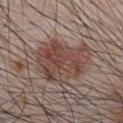No biopsy was performed on this lesion — it was imaged during a full skin examination and was not determined to be concerning.
Cropped from a whole-body photographic skin survey; the tile spans about 15 mm.
Located on the chest.
About 6.5 mm across.
A male subject about 40 years old.
The tile uses white-light illumination.